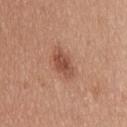Captured during whole-body skin photography for melanoma surveillance; the lesion was not biopsied. Measured at roughly 3.5 mm in maximum diameter. A lesion tile, about 15 mm wide, cut from a 3D total-body photograph. A male patient roughly 35 years of age. The lesion is on the upper back.